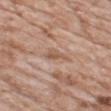workup=catalogued during a skin exam; not biopsied
site=the chest
illumination=white-light
image source=~15 mm crop, total-body skin-cancer survey
diameter=≈3 mm
patient=male, in their mid- to late 70s